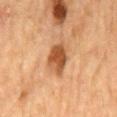Part of a total-body skin-imaging series; this lesion was reviewed on a skin check and was not flagged for biopsy. Automated tile analysis of the lesion measured about 12 CIELAB-L* units darker than the surrounding skin and a normalized border contrast of about 9. And it measured a nevus-likeness score of about 90/100 and lesion-presence confidence of about 100/100. Located on the mid back. A lesion tile, about 15 mm wide, cut from a 3D total-body photograph. A male subject, in their mid- to late 80s or older.